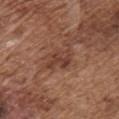{"patient": {"sex": "male", "age_approx": 75}, "site": "chest", "image": {"source": "total-body photography crop", "field_of_view_mm": 15}}